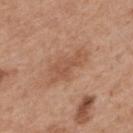No biopsy was performed on this lesion — it was imaged during a full skin examination and was not determined to be concerning.
On the back.
The patient is a female aged 38–42.
A roughly 15 mm field-of-view crop from a total-body skin photograph.